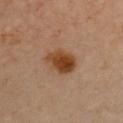Assessment: Part of a total-body skin-imaging series; this lesion was reviewed on a skin check and was not flagged for biopsy. Clinical summary: The lesion is located on the chest. Captured under cross-polarized illumination. The lesion's longest dimension is about 4 mm. Cropped from a whole-body photographic skin survey; the tile spans about 15 mm. Automated image analysis of the tile measured a border-irregularity index near 1.5/10 and a within-lesion color-variation index near 5.5/10. A female subject in their mid- to late 40s.